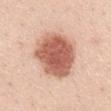This lesion was catalogued during total-body skin photography and was not selected for biopsy. The total-body-photography lesion software estimated a color-variation rating of about 4.5/10. And it measured a classifier nevus-likeness of about 100/100 and lesion-presence confidence of about 100/100. A female patient, aged 38 to 42. This image is a 15 mm lesion crop taken from a total-body photograph. The lesion is located on the mid back.